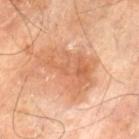biopsy_status: not biopsied; imaged during a skin examination
patient:
  sex: male
  age_approx: 70
image:
  source: total-body photography crop
  field_of_view_mm: 15
lesion_size:
  long_diameter_mm_approx: 7.5
lighting: cross-polarized
site: left thigh
automated_metrics:
  area_mm2_approx: 24.0
  eccentricity: 0.8
  shape_asymmetry: 0.4
  border_irregularity_0_10: 5.0
  color_variation_0_10: 4.5
  peripheral_color_asymmetry: 1.5
  nevus_likeness_0_100: 0
  lesion_detection_confidence_0_100: 100